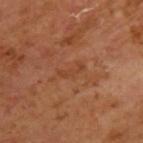This lesion was catalogued during total-body skin photography and was not selected for biopsy. The lesion-visualizer software estimated a mean CIELAB color near L≈41 a*≈24 b*≈33, roughly 5 lightness units darker than nearby skin, and a normalized lesion–skin contrast near 4.5. The software also gave border irregularity of about 7 on a 0–10 scale, a within-lesion color-variation index near 0/10, and a peripheral color-asymmetry measure near 0. Captured under cross-polarized illumination. About 3 mm across. A male patient approximately 65 years of age. On the upper back. A 15 mm crop from a total-body photograph taken for skin-cancer surveillance.